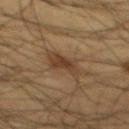Captured during whole-body skin photography for melanoma surveillance; the lesion was not biopsied.
Located on the abdomen.
Measured at roughly 4 mm in maximum diameter.
The lesion-visualizer software estimated a footprint of about 8.5 mm² and two-axis asymmetry of about 0.3. And it measured a border-irregularity index near 4.5/10, internal color variation of about 3 on a 0–10 scale, and peripheral color asymmetry of about 1.
A male patient, aged 58 to 62.
A 15 mm crop from a total-body photograph taken for skin-cancer surveillance.